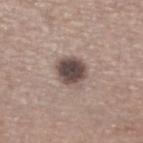Image and clinical context:
Approximately 3.5 mm at its widest. An algorithmic analysis of the crop reported a mean CIELAB color near L≈46 a*≈13 b*≈18, about 16 CIELAB-L* units darker than the surrounding skin, and a lesion-to-skin contrast of about 12 (normalized; higher = more distinct). The software also gave a border-irregularity rating of about 2/10, a color-variation rating of about 4.5/10, and peripheral color asymmetry of about 1. A lesion tile, about 15 mm wide, cut from a 3D total-body photograph. The patient is a male roughly 65 years of age. Imaged with white-light lighting. On the right lower leg.